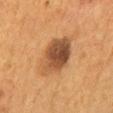notes — no biopsy performed (imaged during a skin exam) | lesion size — ~6.5 mm (longest diameter) | imaging modality — 15 mm crop, total-body photography | image-analysis metrics — a lesion area of about 17 mm² and an eccentricity of roughly 0.85; a border-irregularity index near 2.5/10, internal color variation of about 7 on a 0–10 scale, and radial color variation of about 2.5; a classifier nevus-likeness of about 60/100 and lesion-presence confidence of about 100/100 | subject — female, aged 48–52 | illumination — cross-polarized | anatomic site — the mid back.